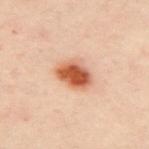biopsy status — no biopsy performed (imaged during a skin exam); imaging modality — ~15 mm crop, total-body skin-cancer survey; subject — female, in their 40s; automated lesion analysis — a mean CIELAB color near L≈58 a*≈27 b*≈36, a lesion–skin lightness drop of about 17, and a normalized lesion–skin contrast near 11; location — the upper back; diameter — about 4 mm.